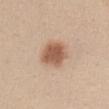Part of a total-body skin-imaging series; this lesion was reviewed on a skin check and was not flagged for biopsy.
The lesion is located on the abdomen.
Cropped from a whole-body photographic skin survey; the tile spans about 15 mm.
Captured under white-light illumination.
A female subject, aged 43 to 47.